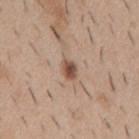No biopsy was performed on this lesion — it was imaged during a full skin examination and was not determined to be concerning. The lesion is on the mid back. Approximately 2.5 mm at its widest. Imaged with white-light lighting. A male subject, about 40 years old. A 15 mm close-up tile from a total-body photography series done for melanoma screening. An algorithmic analysis of the crop reported an eccentricity of roughly 0.8 and two-axis asymmetry of about 0.2. The software also gave border irregularity of about 2 on a 0–10 scale, internal color variation of about 4 on a 0–10 scale, and a peripheral color-asymmetry measure near 1.5. The software also gave an automated nevus-likeness rating near 95 out of 100.